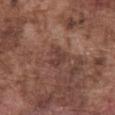{
  "biopsy_status": "not biopsied; imaged during a skin examination",
  "automated_metrics": {
    "cielab_L": 39,
    "cielab_a": 19,
    "cielab_b": 23,
    "vs_skin_contrast_norm": 6.5,
    "border_irregularity_0_10": 4.5,
    "peripheral_color_asymmetry": 0.5,
    "nevus_likeness_0_100": 0
  },
  "lighting": "white-light",
  "patient": {
    "sex": "male",
    "age_approx": 75
  },
  "site": "abdomen",
  "image": {
    "source": "total-body photography crop",
    "field_of_view_mm": 15
  }
}